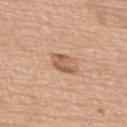biopsy status: no biopsy performed (imaged during a skin exam) | subject: male, approximately 75 years of age | imaging modality: ~15 mm tile from a whole-body skin photo | anatomic site: the upper back | tile lighting: white-light | lesion diameter: ≈3 mm | automated lesion analysis: a border-irregularity rating of about 3/10 and a peripheral color-asymmetry measure near 0.5.